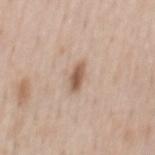The lesion was photographed on a routine skin check and not biopsied; there is no pathology result. A 15 mm crop from a total-body photograph taken for skin-cancer surveillance. The tile uses white-light illumination. A male patient, aged approximately 50. On the mid back. The lesion's longest dimension is about 3 mm.